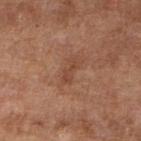Assessment:
This lesion was catalogued during total-body skin photography and was not selected for biopsy.
Image and clinical context:
A 15 mm close-up extracted from a 3D total-body photography capture. The lesion is on the left lower leg. The total-body-photography lesion software estimated a lesion area of about 4 mm², an outline eccentricity of about 0.9 (0 = round, 1 = elongated), and a shape-asymmetry score of about 0.3 (0 = symmetric). And it measured border irregularity of about 4 on a 0–10 scale, internal color variation of about 1 on a 0–10 scale, and radial color variation of about 0. The software also gave a nevus-likeness score of about 0/100 and a detector confidence of about 100 out of 100 that the crop contains a lesion. The subject is a female about 60 years old. Imaged with cross-polarized lighting.